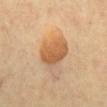patient = roughly 60 years of age; tile lighting = cross-polarized illumination; lesion diameter = ~4.5 mm (longest diameter); image source = ~15 mm tile from a whole-body skin photo; site = the chest.